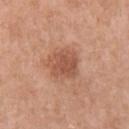imaging modality — ~15 mm crop, total-body skin-cancer survey; location — the right upper arm; subject — female, aged 53 to 57.